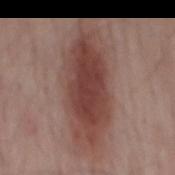Impression: Recorded during total-body skin imaging; not selected for excision or biopsy. Context: This is a white-light tile. The subject is a male roughly 65 years of age. A 15 mm close-up tile from a total-body photography series done for melanoma screening. The lesion-visualizer software estimated a footprint of about 42 mm² and a shape-asymmetry score of about 0.15 (0 = symmetric). It also reported border irregularity of about 3.5 on a 0–10 scale and radial color variation of about 2. The software also gave a nevus-likeness score of about 100/100 and lesion-presence confidence of about 100/100. Measured at roughly 12 mm in maximum diameter. The lesion is located on the mid back.